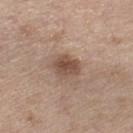Imaged during a routine full-body skin examination; the lesion was not biopsied and no histopathology is available. This image is a 15 mm lesion crop taken from a total-body photograph. Captured under white-light illumination. The subject is a male about 70 years old. The lesion is located on the leg. Approximately 3.5 mm at its widest.